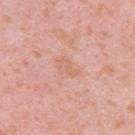The lesion was photographed on a routine skin check and not biopsied; there is no pathology result.
The tile uses white-light illumination.
A 15 mm close-up extracted from a 3D total-body photography capture.
The patient is a female aged approximately 40.
Located on the left upper arm.